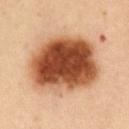lighting = cross-polarized | subject = female, aged around 50 | anatomic site = the abdomen | size = ~8.5 mm (longest diameter) | image source = ~15 mm tile from a whole-body skin photo.